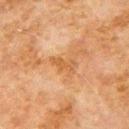The lesion was tiled from a total-body skin photograph and was not biopsied. A male subject aged 78–82. On the left upper arm. Imaged with cross-polarized lighting. A roughly 15 mm field-of-view crop from a total-body skin photograph. Measured at roughly 3.5 mm in maximum diameter.